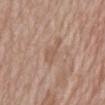patient: female, aged approximately 75
image: 15 mm crop, total-body photography
location: the mid back
illumination: white-light